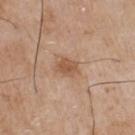A male subject roughly 65 years of age.
On the chest.
An algorithmic analysis of the crop reported a footprint of about 4.5 mm², an eccentricity of roughly 0.65, and a shape-asymmetry score of about 0.2 (0 = symmetric). And it measured a border-irregularity index near 2/10, a within-lesion color-variation index near 2/10, and radial color variation of about 0.5.
A 15 mm close-up tile from a total-body photography series done for melanoma screening.